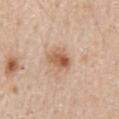This lesion was catalogued during total-body skin photography and was not selected for biopsy. The tile uses white-light illumination. From the back. The patient is a male in their 60s. Measured at roughly 3 mm in maximum diameter. Cropped from a whole-body photographic skin survey; the tile spans about 15 mm.